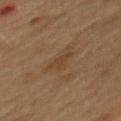The lesion was photographed on a routine skin check and not biopsied; there is no pathology result.
Longest diameter approximately 3.5 mm.
A female subject, in their 60s.
Captured under cross-polarized illumination.
On the mid back.
A 15 mm close-up extracted from a 3D total-body photography capture.
The lesion-visualizer software estimated an outline eccentricity of about 0.85 (0 = round, 1 = elongated). It also reported border irregularity of about 4.5 on a 0–10 scale, internal color variation of about 2 on a 0–10 scale, and radial color variation of about 0.5. It also reported a detector confidence of about 100 out of 100 that the crop contains a lesion.